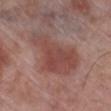{"biopsy_status": "not biopsied; imaged during a skin examination", "patient": {"sex": "male", "age_approx": 70}, "image": {"source": "total-body photography crop", "field_of_view_mm": 15}, "site": "right lower leg", "automated_metrics": {"cielab_L": 46, "cielab_a": 23, "cielab_b": 24, "vs_skin_contrast_norm": 7.0}}